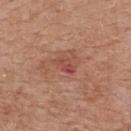<tbp_lesion>
  <biopsy_status>not biopsied; imaged during a skin examination</biopsy_status>
  <automated_metrics>
    <area_mm2_approx>3.5</area_mm2_approx>
    <eccentricity>0.8</eccentricity>
    <shape_asymmetry>0.25</shape_asymmetry>
    <nevus_likeness_0_100>0</nevus_likeness_0_100>
    <lesion_detection_confidence_0_100>100</lesion_detection_confidence_0_100>
  </automated_metrics>
  <patient>
    <sex>female</sex>
    <age_approx>75</age_approx>
  </patient>
  <site>back</site>
  <lesion_size>
    <long_diameter_mm_approx>2.5</long_diameter_mm_approx>
  </lesion_size>
  <lighting>white-light</lighting>
  <image>
    <source>total-body photography crop</source>
    <field_of_view_mm>15</field_of_view_mm>
  </image>
</tbp_lesion>